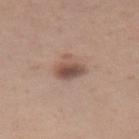tile lighting = white-light illumination
subject = female, aged 28 to 32
image source = total-body-photography crop, ~15 mm field of view
site = the left thigh
lesion diameter = ≈3 mm